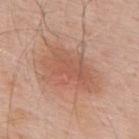The lesion was photographed on a routine skin check and not biopsied; there is no pathology result. The patient is a male aged 53 to 57. An algorithmic analysis of the crop reported a lesion color around L≈57 a*≈23 b*≈30 in CIELAB and a lesion-to-skin contrast of about 5.5 (normalized; higher = more distinct). A 15 mm close-up extracted from a 3D total-body photography capture. From the upper back. Captured under white-light illumination.